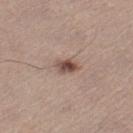image = 15 mm crop, total-body photography | site = the right lower leg | lighting = white-light illumination | lesion diameter = ≈2.5 mm | subject = female, aged 63–67.